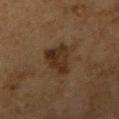Impression: Imaged during a routine full-body skin examination; the lesion was not biopsied and no histopathology is available. Image and clinical context: A female subject approximately 60 years of age. The lesion is on the right upper arm. This image is a 15 mm lesion crop taken from a total-body photograph. Approximately 5 mm at its widest. An algorithmic analysis of the crop reported an outline eccentricity of about 0.75 (0 = round, 1 = elongated) and a shape-asymmetry score of about 0.4 (0 = symmetric). The analysis additionally found a border-irregularity rating of about 4.5/10, a color-variation rating of about 4/10, and peripheral color asymmetry of about 1. It also reported a nevus-likeness score of about 25/100.